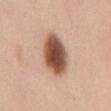follow-up: catalogued during a skin exam; not biopsied | illumination: white-light illumination | patient: female, aged around 35 | automated metrics: a footprint of about 16 mm² and an eccentricity of roughly 0.8; an average lesion color of about L≈52 a*≈21 b*≈29 (CIELAB) and a lesion–skin lightness drop of about 20 | acquisition: total-body-photography crop, ~15 mm field of view | site: the chest | lesion diameter: ~5.5 mm (longest diameter).